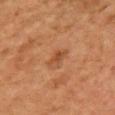follow-up: imaged on a skin check; not biopsied
site: the front of the torso
TBP lesion metrics: a footprint of about 2.5 mm², a shape eccentricity near 0.85, and a symmetry-axis asymmetry near 0.3
imaging modality: 15 mm crop, total-body photography
size: ≈2.5 mm
lighting: cross-polarized illumination
subject: female, aged 58–62